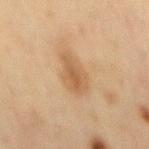notes: catalogued during a skin exam; not biopsied